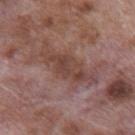| key | value |
|---|---|
| notes | imaged on a skin check; not biopsied |
| TBP lesion metrics | a lesion area of about 14 mm², a shape eccentricity near 0.95, and a symmetry-axis asymmetry near 0.35; about 8 CIELAB-L* units darker than the surrounding skin and a lesion-to-skin contrast of about 6.5 (normalized; higher = more distinct); a border-irregularity rating of about 6/10 and a within-lesion color-variation index near 3.5/10; an automated nevus-likeness rating near 0 out of 100 and a detector confidence of about 60 out of 100 that the crop contains a lesion |
| imaging modality | ~15 mm crop, total-body skin-cancer survey |
| patient | male, aged around 70 |
| anatomic site | the back |